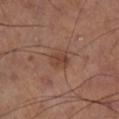{"site": "right thigh", "lesion_size": {"long_diameter_mm_approx": 2.5}, "automated_metrics": {"cielab_L": 43, "cielab_a": 22, "cielab_b": 27, "vs_skin_darker_L": 7.0, "vs_skin_contrast_norm": 6.0}, "image": {"source": "total-body photography crop", "field_of_view_mm": 15}, "lighting": "cross-polarized"}